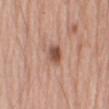biopsy status = catalogued during a skin exam; not biopsied
patient = male, aged approximately 55
tile lighting = white-light illumination
imaging modality = ~15 mm tile from a whole-body skin photo
TBP lesion metrics = a lesion color around L≈52 a*≈22 b*≈29 in CIELAB, a lesion–skin lightness drop of about 13, and a normalized border contrast of about 9; border irregularity of about 2 on a 0–10 scale, a within-lesion color-variation index near 4.5/10, and radial color variation of about 1.5; a nevus-likeness score of about 75/100 and a detector confidence of about 100 out of 100 that the crop contains a lesion
body site = the arm
lesion size = ~2.5 mm (longest diameter)